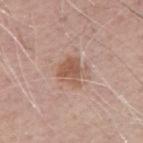Captured during whole-body skin photography for melanoma surveillance; the lesion was not biopsied. The recorded lesion diameter is about 3 mm. A male subject, aged 68–72. Imaged with white-light lighting. A 15 mm crop from a total-body photograph taken for skin-cancer surveillance. From the right upper arm.